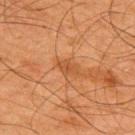follow-up: imaged on a skin check; not biopsied
acquisition: ~15 mm tile from a whole-body skin photo
lesion size: ~2.5 mm (longest diameter)
subject: male, approximately 65 years of age
anatomic site: the back